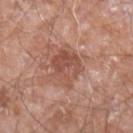Part of a total-body skin-imaging series; this lesion was reviewed on a skin check and was not flagged for biopsy.
A male patient, in their 60s.
From the left forearm.
A close-up tile cropped from a whole-body skin photograph, about 15 mm across.